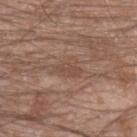Clinical impression: No biopsy was performed on this lesion — it was imaged during a full skin examination and was not determined to be concerning. Acquisition and patient details: On the left forearm. A male subject, aged 18–22. Captured under white-light illumination. Automated tile analysis of the lesion measured border irregularity of about 4.5 on a 0–10 scale, a within-lesion color-variation index near 2/10, and radial color variation of about 0.5. The analysis additionally found a classifier nevus-likeness of about 0/100 and a lesion-detection confidence of about 70/100. A roughly 15 mm field-of-view crop from a total-body skin photograph.